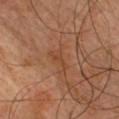Captured under cross-polarized illumination. Automated image analysis of the tile measured an automated nevus-likeness rating near 0 out of 100 and a detector confidence of about 100 out of 100 that the crop contains a lesion. A male subject aged 38 to 42. The lesion is on the front of the torso. A 15 mm close-up extracted from a 3D total-body photography capture. About 3 mm across.